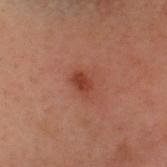Part of a total-body skin-imaging series; this lesion was reviewed on a skin check and was not flagged for biopsy.
A male subject aged 28–32.
This is a cross-polarized tile.
A 15 mm crop from a total-body photograph taken for skin-cancer surveillance.
From the head or neck.
Measured at roughly 3 mm in maximum diameter.